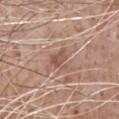workup: catalogued during a skin exam; not biopsied
subject: male, about 65 years old
lighting: white-light illumination
TBP lesion metrics: border irregularity of about 5 on a 0–10 scale, a color-variation rating of about 2.5/10, and a peripheral color-asymmetry measure near 1
acquisition: ~15 mm crop, total-body skin-cancer survey
lesion diameter: about 2.5 mm
body site: the chest